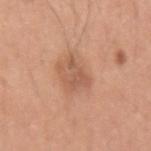{
  "biopsy_status": "not biopsied; imaged during a skin examination",
  "lighting": "white-light",
  "site": "left upper arm",
  "lesion_size": {
    "long_diameter_mm_approx": 3.5
  },
  "patient": {
    "sex": "male",
    "age_approx": 30
  },
  "image": {
    "source": "total-body photography crop",
    "field_of_view_mm": 15
  }
}